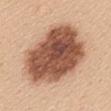Part of a total-body skin-imaging series; this lesion was reviewed on a skin check and was not flagged for biopsy.
Cropped from a total-body skin-imaging series; the visible field is about 15 mm.
From the mid back.
A female patient, in their mid- to late 40s.